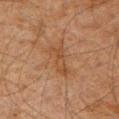Captured during whole-body skin photography for melanoma surveillance; the lesion was not biopsied. Cropped from a total-body skin-imaging series; the visible field is about 15 mm. Automated tile analysis of the lesion measured an eccentricity of roughly 0.9 and a symmetry-axis asymmetry near 0.4. It also reported a mean CIELAB color near L≈36 a*≈17 b*≈28 and roughly 5 lightness units darker than nearby skin. The analysis additionally found border irregularity of about 5 on a 0–10 scale and a within-lesion color-variation index near 3.5/10. And it measured a nevus-likeness score of about 0/100 and a lesion-detection confidence of about 100/100. From the front of the torso. The lesion's longest dimension is about 4 mm. A male patient, aged 58–62.